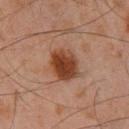Q: Automated lesion metrics?
A: an area of roughly 12 mm², an outline eccentricity of about 0.65 (0 = round, 1 = elongated), and two-axis asymmetry of about 0.1; border irregularity of about 1.5 on a 0–10 scale, internal color variation of about 4.5 on a 0–10 scale, and radial color variation of about 1.5; a nevus-likeness score of about 100/100 and a detector confidence of about 100 out of 100 that the crop contains a lesion
Q: What kind of image is this?
A: ~15 mm tile from a whole-body skin photo
Q: What is the anatomic site?
A: the right lower leg
Q: How large is the lesion?
A: ~4.5 mm (longest diameter)
Q: How was the tile lit?
A: cross-polarized illumination
Q: Who is the patient?
A: male, in their mid- to late 40s